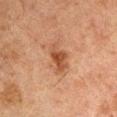<record>
<image>
  <source>total-body photography crop</source>
  <field_of_view_mm>15</field_of_view_mm>
</image>
<lesion_size>
  <long_diameter_mm_approx>4.0</long_diameter_mm_approx>
</lesion_size>
<automated_metrics>
  <peripheral_color_asymmetry>1.0</peripheral_color_asymmetry>
</automated_metrics>
<patient>
  <sex>male</sex>
  <age_approx>50</age_approx>
</patient>
<site>left upper arm</site>
</record>